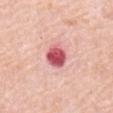{
  "biopsy_status": "not biopsied; imaged during a skin examination",
  "site": "mid back",
  "lighting": "white-light",
  "automated_metrics": {
    "area_mm2_approx": 7.0,
    "eccentricity": 0.6,
    "shape_asymmetry": 0.15,
    "border_irregularity_0_10": 1.5,
    "color_variation_0_10": 6.5
  },
  "patient": {
    "sex": "male",
    "age_approx": 80
  },
  "image": {
    "source": "total-body photography crop",
    "field_of_view_mm": 15
  }
}